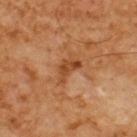Part of a total-body skin-imaging series; this lesion was reviewed on a skin check and was not flagged for biopsy. A male subject, in their 60s. The lesion's longest dimension is about 3.5 mm. A 15 mm crop from a total-body photograph taken for skin-cancer surveillance. Captured under cross-polarized illumination. The lesion is on the upper back. An algorithmic analysis of the crop reported a lesion area of about 4.5 mm², an outline eccentricity of about 0.85 (0 = round, 1 = elongated), and a symmetry-axis asymmetry near 0.55. The analysis additionally found about 9 CIELAB-L* units darker than the surrounding skin. It also reported an automated nevus-likeness rating near 0 out of 100 and lesion-presence confidence of about 100/100.